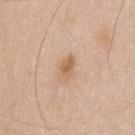Impression: The lesion was photographed on a routine skin check and not biopsied; there is no pathology result. Context: Imaged with white-light lighting. The lesion's longest dimension is about 2.5 mm. A male subject roughly 35 years of age. A 15 mm close-up tile from a total-body photography series done for melanoma screening. The total-body-photography lesion software estimated a shape eccentricity near 0.75 and a symmetry-axis asymmetry near 0.3. And it measured a lesion color around L≈62 a*≈19 b*≈34 in CIELAB. Located on the upper back.